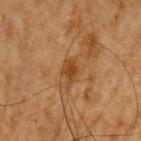<record>
  <biopsy_status>not biopsied; imaged during a skin examination</biopsy_status>
  <patient>
    <sex>male</sex>
    <age_approx>65</age_approx>
  </patient>
  <image>
    <source>total-body photography crop</source>
    <field_of_view_mm>15</field_of_view_mm>
  </image>
  <site>left upper arm</site>
</record>